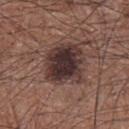This lesion was catalogued during total-body skin photography and was not selected for biopsy.
The patient is a male aged 73 to 77.
The lesion is on the chest.
About 5 mm across.
A region of skin cropped from a whole-body photographic capture, roughly 15 mm wide.
Imaged with white-light lighting.